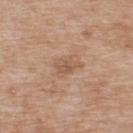<record>
  <image>
    <source>total-body photography crop</source>
    <field_of_view_mm>15</field_of_view_mm>
  </image>
  <lesion_size>
    <long_diameter_mm_approx>3.0</long_diameter_mm_approx>
  </lesion_size>
  <patient>
    <sex>male</sex>
    <age_approx>50</age_approx>
  </patient>
  <site>upper back</site>
  <lighting>white-light</lighting>
</record>